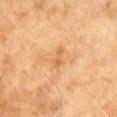| feature | finding |
|---|---|
| biopsy status | total-body-photography surveillance lesion; no biopsy |
| diameter | about 3 mm |
| patient | male, aged 58–62 |
| acquisition | total-body-photography crop, ~15 mm field of view |
| lighting | cross-polarized |
| anatomic site | the back |